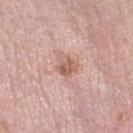The lesion was tiled from a total-body skin photograph and was not biopsied.
The tile uses white-light illumination.
Measured at roughly 2.5 mm in maximum diameter.
A close-up tile cropped from a whole-body skin photograph, about 15 mm across.
The patient is a female approximately 50 years of age.
The lesion is on the right lower leg.
The total-body-photography lesion software estimated a classifier nevus-likeness of about 20/100 and a lesion-detection confidence of about 100/100.